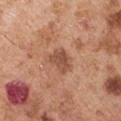Q: Is there a histopathology result?
A: imaged on a skin check; not biopsied
Q: Who is the patient?
A: male, in their mid- to late 50s
Q: What lighting was used for the tile?
A: white-light illumination
Q: What is the imaging modality?
A: ~15 mm crop, total-body skin-cancer survey
Q: How large is the lesion?
A: about 3.5 mm
Q: What is the anatomic site?
A: the left upper arm